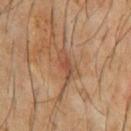Impression: Captured during whole-body skin photography for melanoma surveillance; the lesion was not biopsied. Background: The lesion's longest dimension is about 4 mm. A male subject, approximately 60 years of age. The lesion-visualizer software estimated an outline eccentricity of about 0.9 (0 = round, 1 = elongated) and a symmetry-axis asymmetry near 0.45. The analysis additionally found an average lesion color of about L≈45 a*≈21 b*≈29 (CIELAB), roughly 8 lightness units darker than nearby skin, and a normalized border contrast of about 6.5. The software also gave border irregularity of about 5 on a 0–10 scale, internal color variation of about 2.5 on a 0–10 scale, and radial color variation of about 0.5. It also reported an automated nevus-likeness rating near 0 out of 100. The lesion is on the chest. A close-up tile cropped from a whole-body skin photograph, about 15 mm across. This is a cross-polarized tile.